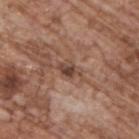Notes:
• notes: no biopsy performed (imaged during a skin exam)
• illumination: white-light illumination
• image-analysis metrics: an automated nevus-likeness rating near 0 out of 100
• subject: male, aged 68–72
• anatomic site: the upper back
• lesion diameter: ~2.5 mm (longest diameter)
• image source: ~15 mm crop, total-body skin-cancer survey